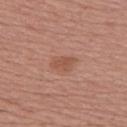A 15 mm crop from a total-body photograph taken for skin-cancer surveillance. On the upper back. About 3 mm across. A female subject, aged approximately 50. Automated tile analysis of the lesion measured a lesion area of about 4.5 mm² and a shape-asymmetry score of about 0.3 (0 = symmetric). The software also gave an average lesion color of about L≈52 a*≈23 b*≈30 (CIELAB) and a lesion-to-skin contrast of about 6 (normalized; higher = more distinct). It also reported border irregularity of about 3 on a 0–10 scale. And it measured a classifier nevus-likeness of about 35/100. Captured under white-light illumination.